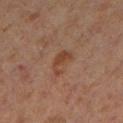No biopsy was performed on this lesion — it was imaged during a full skin examination and was not determined to be concerning. Measured at roughly 3.5 mm in maximum diameter. On the leg. A lesion tile, about 15 mm wide, cut from a 3D total-body photograph. A male subject aged 58 to 62. Imaged with cross-polarized lighting. The total-body-photography lesion software estimated a lesion area of about 4.5 mm², a shape eccentricity near 0.85, and a symmetry-axis asymmetry near 0.5. And it measured lesion-presence confidence of about 100/100.